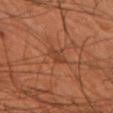• lesion size · ~2.5 mm (longest diameter)
• image-analysis metrics · a mean CIELAB color near L≈31 a*≈20 b*≈26, a lesion–skin lightness drop of about 5, and a normalized lesion–skin contrast near 5; internal color variation of about 1 on a 0–10 scale
• acquisition · total-body-photography crop, ~15 mm field of view
• tile lighting · cross-polarized
• patient · male, aged 58–62
• site · the left forearm